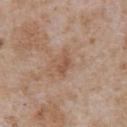The lesion was photographed on a routine skin check and not biopsied; there is no pathology result. Captured under white-light illumination. The lesion-visualizer software estimated a border-irregularity index near 3/10. A 15 mm crop from a total-body photograph taken for skin-cancer surveillance. Measured at roughly 3 mm in maximum diameter. The lesion is on the chest. A male patient, about 65 years old.